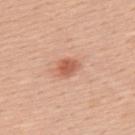Impression:
The lesion was photographed on a routine skin check and not biopsied; there is no pathology result.
Acquisition and patient details:
A 15 mm close-up tile from a total-body photography series done for melanoma screening. The patient is a male aged approximately 55. Located on the upper back.